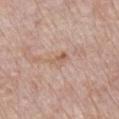From the chest. The total-body-photography lesion software estimated a border-irregularity index near 3.5/10, internal color variation of about 3.5 on a 0–10 scale, and peripheral color asymmetry of about 1. The software also gave a classifier nevus-likeness of about 0/100 and lesion-presence confidence of about 100/100. The recorded lesion diameter is about 2.5 mm. A close-up tile cropped from a whole-body skin photograph, about 15 mm across. The patient is a male aged approximately 70.